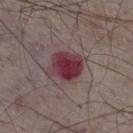Clinical impression:
This lesion was catalogued during total-body skin photography and was not selected for biopsy.
Image and clinical context:
The lesion's longest dimension is about 4 mm. A 15 mm close-up tile from a total-body photography series done for melanoma screening. A male subject, roughly 75 years of age. The total-body-photography lesion software estimated an area of roughly 11 mm² and two-axis asymmetry of about 0.2. And it measured about 13 CIELAB-L* units darker than the surrounding skin and a normalized lesion–skin contrast near 11.5. It also reported a border-irregularity rating of about 2.5/10 and peripheral color asymmetry of about 1.5. It also reported a classifier nevus-likeness of about 0/100 and a detector confidence of about 100 out of 100 that the crop contains a lesion. Located on the right thigh. Imaged with white-light lighting.